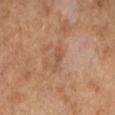Clinical impression: Imaged during a routine full-body skin examination; the lesion was not biopsied and no histopathology is available. Acquisition and patient details: A lesion tile, about 15 mm wide, cut from a 3D total-body photograph. Imaged with cross-polarized lighting. Measured at roughly 3 mm in maximum diameter. The subject is a male roughly 65 years of age.